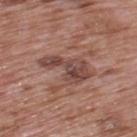Q: Was a biopsy performed?
A: total-body-photography surveillance lesion; no biopsy
Q: How was the tile lit?
A: white-light
Q: What is the lesion's diameter?
A: ≈6 mm
Q: What is the anatomic site?
A: the upper back
Q: How was this image acquired?
A: ~15 mm tile from a whole-body skin photo
Q: Automated lesion metrics?
A: a footprint of about 11 mm² and an eccentricity of roughly 0.85; a classifier nevus-likeness of about 0/100 and a lesion-detection confidence of about 100/100
Q: Who is the patient?
A: male, approximately 70 years of age